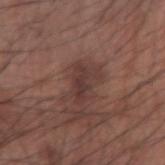follow-up: imaged on a skin check; not biopsied | anatomic site: the left forearm | automated lesion analysis: a lesion color around L≈36 a*≈19 b*≈20 in CIELAB, a lesion–skin lightness drop of about 8, and a lesion-to-skin contrast of about 7.5 (normalized; higher = more distinct); border irregularity of about 6 on a 0–10 scale, internal color variation of about 2.5 on a 0–10 scale, and radial color variation of about 1; a classifier nevus-likeness of about 10/100 and a detector confidence of about 100 out of 100 that the crop contains a lesion | imaging modality: 15 mm crop, total-body photography | lesion diameter: about 4 mm | subject: male, approximately 75 years of age.